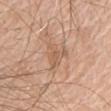Recorded during total-body skin imaging; not selected for excision or biopsy.